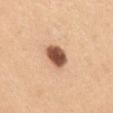Clinical impression: Captured during whole-body skin photography for melanoma surveillance; the lesion was not biopsied. Acquisition and patient details: On the mid back. The tile uses white-light illumination. Cropped from a whole-body photographic skin survey; the tile spans about 15 mm. A female patient, aged approximately 30.